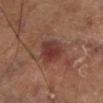Acquisition and patient details:
On the left lower leg. Imaged with cross-polarized lighting. A male patient, approximately 70 years of age. A lesion tile, about 15 mm wide, cut from a 3D total-body photograph.